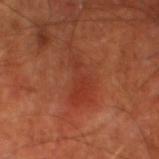Imaged during a routine full-body skin examination; the lesion was not biopsied and no histopathology is available.
The recorded lesion diameter is about 6 mm.
A male patient roughly 70 years of age.
The lesion is located on the left thigh.
Captured under cross-polarized illumination.
A 15 mm crop from a total-body photograph taken for skin-cancer surveillance.